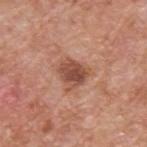Assessment: No biopsy was performed on this lesion — it was imaged during a full skin examination and was not determined to be concerning. Acquisition and patient details: The patient is a male aged 58 to 62. Longest diameter approximately 3 mm. A close-up tile cropped from a whole-body skin photograph, about 15 mm across. On the back.